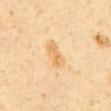Captured during whole-body skin photography for melanoma surveillance; the lesion was not biopsied. On the abdomen. Approximately 3.5 mm at its widest. The subject is a male in their 60s. Imaged with cross-polarized lighting. This image is a 15 mm lesion crop taken from a total-body photograph. An algorithmic analysis of the crop reported an area of roughly 6 mm² and an eccentricity of roughly 0.85. The software also gave a classifier nevus-likeness of about 5/100 and a detector confidence of about 100 out of 100 that the crop contains a lesion.